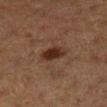No biopsy was performed on this lesion — it was imaged during a full skin examination and was not determined to be concerning.
The total-body-photography lesion software estimated a classifier nevus-likeness of about 95/100 and lesion-presence confidence of about 100/100.
The lesion's longest dimension is about 3 mm.
A female patient, about 40 years old.
A 15 mm close-up tile from a total-body photography series done for melanoma screening.
Located on the right lower leg.